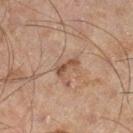This lesion was catalogued during total-body skin photography and was not selected for biopsy.